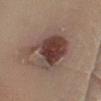Part of a total-body skin-imaging series; this lesion was reviewed on a skin check and was not flagged for biopsy.
Imaged with white-light lighting.
Longest diameter approximately 6.5 mm.
Located on the leg.
Automated image analysis of the tile measured a footprint of about 20 mm², an outline eccentricity of about 0.6 (0 = round, 1 = elongated), and two-axis asymmetry of about 0.4. It also reported a lesion color around L≈41 a*≈18 b*≈22 in CIELAB, a lesion–skin lightness drop of about 14, and a lesion-to-skin contrast of about 11 (normalized; higher = more distinct).
A 15 mm close-up extracted from a 3D total-body photography capture.
A female subject aged 53–57.